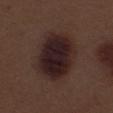Q: Is there a histopathology result?
A: imaged on a skin check; not biopsied
Q: What did automated image analysis measure?
A: an eccentricity of roughly 0.65 and a shape-asymmetry score of about 0.15 (0 = symmetric); an average lesion color of about L≈22 a*≈15 b*≈15 (CIELAB) and a normalized border contrast of about 13; border irregularity of about 1.5 on a 0–10 scale and internal color variation of about 5.5 on a 0–10 scale; a detector confidence of about 100 out of 100 that the crop contains a lesion
Q: What is the imaging modality?
A: 15 mm crop, total-body photography
Q: What lighting was used for the tile?
A: white-light
Q: Who is the patient?
A: male, aged 68–72
Q: Lesion size?
A: about 6.5 mm
Q: Where on the body is the lesion?
A: the left thigh